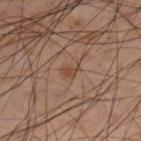No biopsy was performed on this lesion — it was imaged during a full skin examination and was not determined to be concerning. Longest diameter approximately 2.5 mm. A close-up tile cropped from a whole-body skin photograph, about 15 mm across. The total-body-photography lesion software estimated a mean CIELAB color near L≈46 a*≈19 b*≈29 and a lesion–skin lightness drop of about 7. The software also gave a border-irregularity rating of about 4.5/10, a within-lesion color-variation index near 1/10, and radial color variation of about 0.5. The analysis additionally found a nevus-likeness score of about 10/100. A male subject, aged 53–57. Imaged with cross-polarized lighting. The lesion is located on the right lower leg.